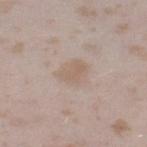No biopsy was performed on this lesion — it was imaged during a full skin examination and was not determined to be concerning.
The lesion is on the right thigh.
A female subject aged approximately 25.
A region of skin cropped from a whole-body photographic capture, roughly 15 mm wide.
Captured under white-light illumination.